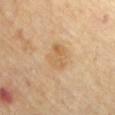<case>
<biopsy_status>not biopsied; imaged during a skin examination</biopsy_status>
<site>chest</site>
<automated_metrics>
  <nevus_likeness_0_100>5</nevus_likeness_0_100>
</automated_metrics>
<patient>
  <sex>female</sex>
  <age_approx>70</age_approx>
</patient>
<lesion_size>
  <long_diameter_mm_approx>3.5</long_diameter_mm_approx>
</lesion_size>
<lighting>cross-polarized</lighting>
<image>
  <source>total-body photography crop</source>
  <field_of_view_mm>15</field_of_view_mm>
</image>
</case>